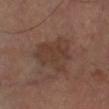notes — no biopsy performed (imaged during a skin exam) | image-analysis metrics — a shape-asymmetry score of about 0.4 (0 = symmetric); an average lesion color of about L≈36 a*≈17 b*≈24 (CIELAB) and a lesion-to-skin contrast of about 6 (normalized; higher = more distinct); border irregularity of about 4.5 on a 0–10 scale, internal color variation of about 2.5 on a 0–10 scale, and peripheral color asymmetry of about 1; a classifier nevus-likeness of about 0/100 and a detector confidence of about 100 out of 100 that the crop contains a lesion | site — the leg | image source — ~15 mm tile from a whole-body skin photo | tile lighting — cross-polarized illumination | diameter — about 5.5 mm | subject — approximately 65 years of age.